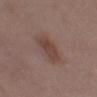The lesion was tiled from a total-body skin photograph and was not biopsied. A female subject, in their mid- to late 30s. A region of skin cropped from a whole-body photographic capture, roughly 15 mm wide. This is a white-light tile. Automated tile analysis of the lesion measured a footprint of about 9.5 mm², an eccentricity of roughly 0.95, and a shape-asymmetry score of about 0.25 (0 = symmetric). The software also gave a mean CIELAB color near L≈43 a*≈18 b*≈23. The lesion is located on the abdomen. The recorded lesion diameter is about 5.5 mm.